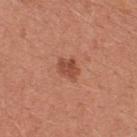  biopsy_status: not biopsied; imaged during a skin examination
  image:
    source: total-body photography crop
    field_of_view_mm: 15
  site: front of the torso
  automated_metrics:
    area_mm2_approx: 4.5
    eccentricity: 0.65
    shape_asymmetry: 0.25
    cielab_L: 49
    cielab_a: 27
    cielab_b: 32
    vs_skin_darker_L: 10.0
    vs_skin_contrast_norm: 7.5
    border_irregularity_0_10: 2.5
    color_variation_0_10: 3.0
    peripheral_color_asymmetry: 1.0
  lighting: white-light
  lesion_size:
    long_diameter_mm_approx: 2.5
  patient:
    sex: female
    age_approx: 35